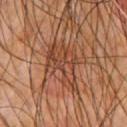image:
  source: total-body photography crop
  field_of_view_mm: 15
automated_metrics:
  shape_asymmetry: 0.4
  cielab_L: 42
  cielab_a: 23
  cielab_b: 31
  border_irregularity_0_10: 5.5
  color_variation_0_10: 6.0
  peripheral_color_asymmetry: 2.5
site: chest
lighting: cross-polarized
lesion_size:
  long_diameter_mm_approx: 6.0
patient:
  sex: male
  age_approx: 65